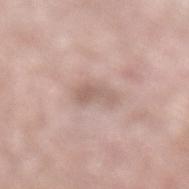patient — male, aged approximately 80
lesion size — ~3 mm (longest diameter)
automated metrics — a footprint of about 4 mm² and an eccentricity of roughly 0.85; a lesion color around L≈60 a*≈17 b*≈24 in CIELAB, a lesion–skin lightness drop of about 9, and a normalized lesion–skin contrast near 6; a border-irregularity rating of about 4.5/10, a within-lesion color-variation index near 1/10, and radial color variation of about 0.5; a classifier nevus-likeness of about 0/100 and a lesion-detection confidence of about 100/100
lighting — white-light illumination
image — total-body-photography crop, ~15 mm field of view
anatomic site — the left lower leg